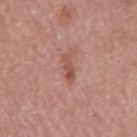This lesion was catalogued during total-body skin photography and was not selected for biopsy.
A male subject, aged approximately 70.
A 15 mm crop from a total-body photograph taken for skin-cancer surveillance.
Measured at roughly 3 mm in maximum diameter.
The tile uses white-light illumination.
Automated tile analysis of the lesion measured an area of roughly 3.5 mm², an eccentricity of roughly 0.9, and a shape-asymmetry score of about 0.3 (0 = symmetric). And it measured an automated nevus-likeness rating near 10 out of 100 and a detector confidence of about 100 out of 100 that the crop contains a lesion.
The lesion is located on the mid back.